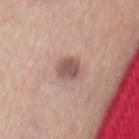follow-up: catalogued during a skin exam; not biopsied
imaging modality: ~15 mm tile from a whole-body skin photo
patient: male, roughly 55 years of age
illumination: white-light
body site: the mid back